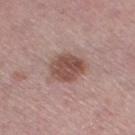<record>
<biopsy_status>not biopsied; imaged during a skin examination</biopsy_status>
<lighting>white-light</lighting>
<lesion_size>
  <long_diameter_mm_approx>4.0</long_diameter_mm_approx>
</lesion_size>
<site>right thigh</site>
<image>
  <source>total-body photography crop</source>
  <field_of_view_mm>15</field_of_view_mm>
</image>
<patient>
  <sex>female</sex>
  <age_approx>50</age_approx>
</patient>
<automated_metrics>
  <area_mm2_approx>10.0</area_mm2_approx>
  <eccentricity>0.5</eccentricity>
  <shape_asymmetry>0.15</shape_asymmetry>
  <color_variation_0_10>4.0</color_variation_0_10>
  <peripheral_color_asymmetry>1.5</peripheral_color_asymmetry>
</automated_metrics>
</record>